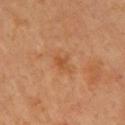Assessment:
No biopsy was performed on this lesion — it was imaged during a full skin examination and was not determined to be concerning.
Acquisition and patient details:
The tile uses cross-polarized illumination. On the upper back. The total-body-photography lesion software estimated a normalized border contrast of about 6. It also reported a border-irregularity rating of about 3/10 and a peripheral color-asymmetry measure near 0.5. The software also gave an automated nevus-likeness rating near 0 out of 100. The patient is a female roughly 35 years of age. A roughly 15 mm field-of-view crop from a total-body skin photograph.